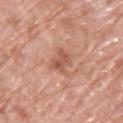biopsy status=imaged on a skin check; not biopsied | location=the chest | image=total-body-photography crop, ~15 mm field of view | patient=male, about 70 years old | lighting=white-light.